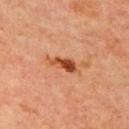biopsy status = total-body-photography surveillance lesion; no biopsy | anatomic site = the chest | diameter = ≈4 mm | patient = male, about 70 years old | image = total-body-photography crop, ~15 mm field of view.